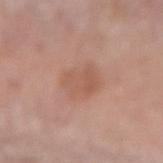The lesion is on the left forearm.
Automated image analysis of the tile measured roughly 6 lightness units darker than nearby skin and a lesion-to-skin contrast of about 5 (normalized; higher = more distinct). It also reported a border-irregularity index near 2/10 and peripheral color asymmetry of about 1.
The subject is a male aged around 70.
Cropped from a whole-body photographic skin survey; the tile spans about 15 mm.